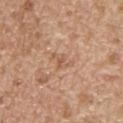Assessment:
The lesion was tiled from a total-body skin photograph and was not biopsied.
Background:
Captured under white-light illumination. Cropped from a whole-body photographic skin survey; the tile spans about 15 mm. Located on the back. A male patient approximately 70 years of age.